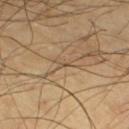Clinical impression:
The lesion was tiled from a total-body skin photograph and was not biopsied.
Acquisition and patient details:
A male subject about 65 years old. A region of skin cropped from a whole-body photographic capture, roughly 15 mm wide. Imaged with cross-polarized lighting. Approximately 1 mm at its widest. On the left lower leg.